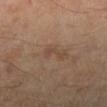biopsy_status: not biopsied; imaged during a skin examination
image:
  source: total-body photography crop
  field_of_view_mm: 15
lighting: cross-polarized
patient:
  sex: male
  age_approx: 55
lesion_size:
  long_diameter_mm_approx: 3.0
site: leg
automated_metrics:
  cielab_L: 43
  cielab_a: 18
  cielab_b: 27
  vs_skin_darker_L: 6.0
  vs_skin_contrast_norm: 5.0
  nevus_likeness_0_100: 0
  lesion_detection_confidence_0_100: 100